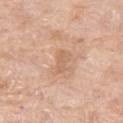* biopsy status · imaged on a skin check; not biopsied
* image · ~15 mm crop, total-body skin-cancer survey
* anatomic site · the left thigh
* patient · female, aged 73–77
* lesion size · about 2.5 mm
* lighting · white-light illumination